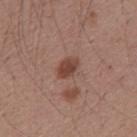The lesion was tiled from a total-body skin photograph and was not biopsied.
Approximately 3 mm at its widest.
The lesion is located on the mid back.
A male subject in their mid- to late 50s.
The lesion-visualizer software estimated an area of roughly 5 mm². The software also gave an average lesion color of about L≈44 a*≈21 b*≈26 (CIELAB), a lesion–skin lightness drop of about 11, and a normalized lesion–skin contrast near 8.5.
A roughly 15 mm field-of-view crop from a total-body skin photograph.